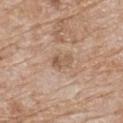| field | value |
|---|---|
| notes | total-body-photography surveillance lesion; no biopsy |
| illumination | white-light illumination |
| location | the upper back |
| lesion size | ≈2.5 mm |
| image source | ~15 mm crop, total-body skin-cancer survey |
| subject | female, about 85 years old or older |